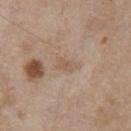Q: Was a biopsy performed?
A: total-body-photography surveillance lesion; no biopsy
Q: How was this image acquired?
A: total-body-photography crop, ~15 mm field of view
Q: What did automated image analysis measure?
A: a lesion area of about 3.5 mm², a shape eccentricity near 0.8, and a shape-asymmetry score of about 0.3 (0 = symmetric); a mean CIELAB color near L≈56 a*≈15 b*≈29; a border-irregularity index near 3/10 and a within-lesion color-variation index near 2.5/10; lesion-presence confidence of about 100/100
Q: Who is the patient?
A: male, aged 63–67
Q: Where on the body is the lesion?
A: the chest